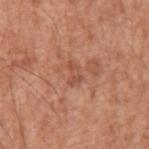No biopsy was performed on this lesion — it was imaged during a full skin examination and was not determined to be concerning. A roughly 15 mm field-of-view crop from a total-body skin photograph. About 2.5 mm across. Automated tile analysis of the lesion measured an eccentricity of roughly 0.8 and two-axis asymmetry of about 0.45. Imaged with white-light lighting. On the arm. The subject is a male aged approximately 65.